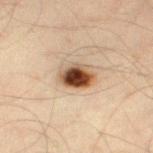biopsy status: total-body-photography surveillance lesion; no biopsy | automated lesion analysis: an area of roughly 8.5 mm² and an eccentricity of roughly 0.75; a mean CIELAB color near L≈42 a*≈17 b*≈29, about 19 CIELAB-L* units darker than the surrounding skin, and a lesion-to-skin contrast of about 14 (normalized; higher = more distinct); an automated nevus-likeness rating near 100 out of 100 and a detector confidence of about 100 out of 100 that the crop contains a lesion | lighting: cross-polarized illumination | anatomic site: the leg | acquisition: 15 mm crop, total-body photography | patient: male, approximately 45 years of age.